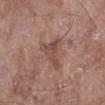Captured during whole-body skin photography for melanoma surveillance; the lesion was not biopsied.
The subject is a female in their mid- to late 70s.
A 15 mm close-up tile from a total-body photography series done for melanoma screening.
The total-body-photography lesion software estimated a border-irregularity rating of about 6/10 and radial color variation of about 1. It also reported a classifier nevus-likeness of about 0/100.
Longest diameter approximately 4 mm.
Imaged with white-light lighting.
On the right lower leg.